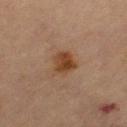Part of a total-body skin-imaging series; this lesion was reviewed on a skin check and was not flagged for biopsy. A 15 mm crop from a total-body photograph taken for skin-cancer surveillance. The subject is a female about 70 years old. From the leg. An algorithmic analysis of the crop reported a lesion area of about 7 mm² and a symmetry-axis asymmetry near 0.3. It also reported internal color variation of about 4 on a 0–10 scale. Imaged with cross-polarized lighting.